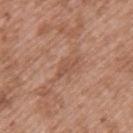Recorded during total-body skin imaging; not selected for excision or biopsy. A male patient about 50 years old. This is a white-light tile. Automated tile analysis of the lesion measured an average lesion color of about L≈52 a*≈22 b*≈30 (CIELAB), about 7 CIELAB-L* units darker than the surrounding skin, and a lesion-to-skin contrast of about 5 (normalized; higher = more distinct). The analysis additionally found border irregularity of about 4 on a 0–10 scale, a within-lesion color-variation index near 1/10, and a peripheral color-asymmetry measure near 0.5. The analysis additionally found an automated nevus-likeness rating near 0 out of 100. The recorded lesion diameter is about 3.5 mm. Located on the upper back. A 15 mm close-up tile from a total-body photography series done for melanoma screening.